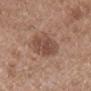workup: no biopsy performed (imaged during a skin exam)
acquisition: ~15 mm crop, total-body skin-cancer survey
anatomic site: the mid back
lesion size: about 5.5 mm
image-analysis metrics: a lesion area of about 12 mm², an outline eccentricity of about 0.8 (0 = round, 1 = elongated), and a symmetry-axis asymmetry near 0.2; a within-lesion color-variation index near 3.5/10 and a peripheral color-asymmetry measure near 1; a lesion-detection confidence of about 100/100
patient: male, in their mid-70s